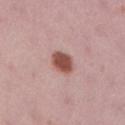Part of a total-body skin-imaging series; this lesion was reviewed on a skin check and was not flagged for biopsy.
Approximately 3 mm at its widest.
Imaged with white-light lighting.
The patient is a female aged 13 to 17.
The lesion is on the right lower leg.
A 15 mm crop from a total-body photograph taken for skin-cancer surveillance.
Automated image analysis of the tile measured an area of roughly 6.5 mm², a shape eccentricity near 0.5, and two-axis asymmetry of about 0.15. The analysis additionally found a lesion color around L≈51 a*≈24 b*≈25 in CIELAB and a lesion–skin lightness drop of about 15. The analysis additionally found border irregularity of about 1 on a 0–10 scale and a color-variation rating of about 2.5/10. It also reported an automated nevus-likeness rating near 100 out of 100.